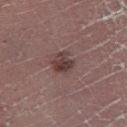| key | value |
|---|---|
| follow-up | no biopsy performed (imaged during a skin exam) |
| illumination | cross-polarized |
| anatomic site | the right lower leg |
| image source | 15 mm crop, total-body photography |
| diameter | ≈2.5 mm |
| patient | male, roughly 50 years of age |